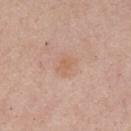Case summary:
- notes · total-body-photography surveillance lesion; no biopsy
- lighting · white-light
- TBP lesion metrics · a mean CIELAB color near L≈62 a*≈19 b*≈31, about 6 CIELAB-L* units darker than the surrounding skin, and a normalized border contrast of about 5; a classifier nevus-likeness of about 0/100 and lesion-presence confidence of about 100/100
- size · ≈2.5 mm
- subject · male, roughly 55 years of age
- image · ~15 mm crop, total-body skin-cancer survey
- site · the mid back